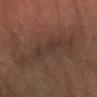<case>
<biopsy_status>not biopsied; imaged during a skin examination</biopsy_status>
<site>left forearm</site>
<patient>
  <sex>male</sex>
  <age_approx>65</age_approx>
</patient>
<lesion_size>
  <long_diameter_mm_approx>4.5</long_diameter_mm_approx>
</lesion_size>
<image>
  <source>total-body photography crop</source>
  <field_of_view_mm>15</field_of_view_mm>
</image>
<lighting>cross-polarized</lighting>
</case>